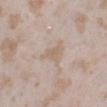Impression: The lesion was photographed on a routine skin check and not biopsied; there is no pathology result.